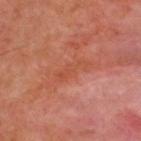Clinical impression: This lesion was catalogued during total-body skin photography and was not selected for biopsy. Acquisition and patient details: On the upper back. A male patient, roughly 50 years of age. Measured at roughly 4.5 mm in maximum diameter. An algorithmic analysis of the crop reported a footprint of about 5.5 mm² and a shape eccentricity near 0.95. The analysis additionally found a border-irregularity rating of about 4/10, a within-lesion color-variation index near 1.5/10, and peripheral color asymmetry of about 0. A region of skin cropped from a whole-body photographic capture, roughly 15 mm wide.